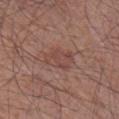| key | value |
|---|---|
| follow-up | catalogued during a skin exam; not biopsied |
| patient | male, in their mid-50s |
| anatomic site | the right lower leg |
| lesion diameter | about 4 mm |
| acquisition | 15 mm crop, total-body photography |